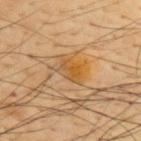Q: Was this lesion biopsied?
A: no biopsy performed (imaged during a skin exam)
Q: What is the anatomic site?
A: the upper back
Q: How was this image acquired?
A: ~15 mm tile from a whole-body skin photo
Q: What lighting was used for the tile?
A: cross-polarized
Q: Who is the patient?
A: male, in their mid-40s